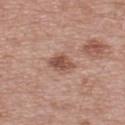follow-up: total-body-photography surveillance lesion; no biopsy | imaging modality: ~15 mm crop, total-body skin-cancer survey | automated metrics: a mean CIELAB color near L≈55 a*≈20 b*≈27, about 9 CIELAB-L* units darker than the surrounding skin, and a normalized border contrast of about 6; border irregularity of about 5 on a 0–10 scale, a within-lesion color-variation index near 5/10, and radial color variation of about 1.5; a lesion-detection confidence of about 100/100 | anatomic site: the upper back | patient: male, aged 63 to 67 | illumination: white-light | diameter: ≈4.5 mm.